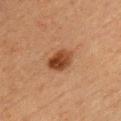Captured during whole-body skin photography for melanoma surveillance; the lesion was not biopsied.
On the front of the torso.
Cropped from a whole-body photographic skin survey; the tile spans about 15 mm.
Measured at roughly 3.5 mm in maximum diameter.
The patient is a female approximately 55 years of age.
Imaged with cross-polarized lighting.
The lesion-visualizer software estimated a border-irregularity rating of about 2/10, a color-variation rating of about 5/10, and peripheral color asymmetry of about 2. The software also gave an automated nevus-likeness rating near 100 out of 100 and a detector confidence of about 100 out of 100 that the crop contains a lesion.